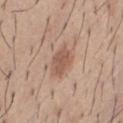Imaged during a routine full-body skin examination; the lesion was not biopsied and no histopathology is available.
About 4 mm across.
Cropped from a whole-body photographic skin survey; the tile spans about 15 mm.
A male subject, aged around 60.
Located on the front of the torso.
The tile uses white-light illumination.